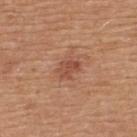notes = imaged on a skin check; not biopsied
illumination = white-light illumination
body site = the upper back
automated lesion analysis = a lesion-detection confidence of about 100/100
diameter = about 3 mm
imaging modality = ~15 mm crop, total-body skin-cancer survey
subject = male, aged around 65